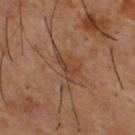biopsy status = catalogued during a skin exam; not biopsied | anatomic site = the upper back | lesion size = about 3 mm | subject = male, aged approximately 55 | automated metrics = a mean CIELAB color near L≈40 a*≈20 b*≈29 and a normalized lesion–skin contrast near 6; internal color variation of about 2.5 on a 0–10 scale and peripheral color asymmetry of about 0.5 | image = 15 mm crop, total-body photography | tile lighting = cross-polarized.